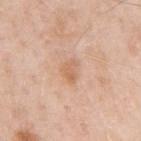biopsy status: total-body-photography surveillance lesion; no biopsy
subject: male, approximately 55 years of age
anatomic site: the left upper arm
image source: ~15 mm crop, total-body skin-cancer survey
automated metrics: an area of roughly 4 mm² and an eccentricity of roughly 0.7